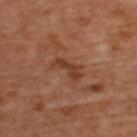Notes:
* follow-up · imaged on a skin check; not biopsied
* acquisition · ~15 mm crop, total-body skin-cancer survey
* lesion size · ≈3.5 mm
* automated lesion analysis · a border-irregularity rating of about 5/10, internal color variation of about 1 on a 0–10 scale, and radial color variation of about 0.5; a lesion-detection confidence of about 100/100
* tile lighting · cross-polarized
* body site · the back
* subject · female, in their mid-40s